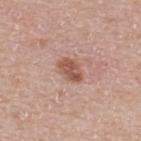<case>
  <biopsy_status>not biopsied; imaged during a skin examination</biopsy_status>
  <lesion_size>
    <long_diameter_mm_approx>3.5</long_diameter_mm_approx>
  </lesion_size>
  <image>
    <source>total-body photography crop</source>
    <field_of_view_mm>15</field_of_view_mm>
  </image>
  <site>upper back</site>
  <patient>
    <sex>female</sex>
    <age_approx>40</age_approx>
  </patient>
</case>